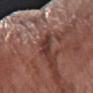  patient:
    sex: female
    age_approx: 60
  lesion_size:
    long_diameter_mm_approx: 3.0
  lighting: white-light
  image:
    source: total-body photography crop
    field_of_view_mm: 15
  site: right forearm